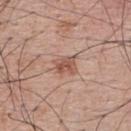{"site": "upper back", "lesion_size": {"long_diameter_mm_approx": 2.5}, "image": {"source": "total-body photography crop", "field_of_view_mm": 15}, "patient": {"sex": "male", "age_approx": 65}, "lighting": "white-light"}